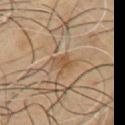This lesion was catalogued during total-body skin photography and was not selected for biopsy. Automated image analysis of the tile measured a classifier nevus-likeness of about 0/100. Captured under cross-polarized illumination. From the front of the torso. The recorded lesion diameter is about 2.5 mm. A male subject aged approximately 65. A 15 mm crop from a total-body photograph taken for skin-cancer surveillance.